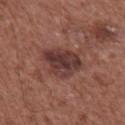workup: no biopsy performed (imaged during a skin exam)
acquisition: ~15 mm tile from a whole-body skin photo
lighting: white-light
anatomic site: the front of the torso
patient: male, aged 73 to 77